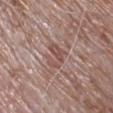  biopsy_status: not biopsied; imaged during a skin examination
  patient:
    sex: male
    age_approx: 65
  site: arm
  lighting: white-light
  image:
    source: total-body photography crop
    field_of_view_mm: 15
  lesion_size:
    long_diameter_mm_approx: 3.5